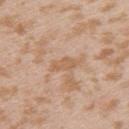Recorded during total-body skin imaging; not selected for excision or biopsy. Automated image analysis of the tile measured a border-irregularity index near 3/10, a color-variation rating of about 1/10, and a peripheral color-asymmetry measure near 0.5. It also reported an automated nevus-likeness rating near 0 out of 100 and a detector confidence of about 100 out of 100 that the crop contains a lesion. On the arm. A 15 mm close-up extracted from a 3D total-body photography capture. Longest diameter approximately 3.5 mm. The subject is a female roughly 25 years of age.